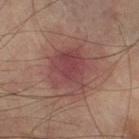This lesion was catalogued during total-body skin photography and was not selected for biopsy.